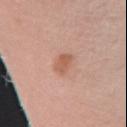Notes:
- subject — female, aged 48–52
- imaging modality — total-body-photography crop, ~15 mm field of view
- site — the left forearm
- lesion diameter — ≈3.5 mm
- lighting — white-light illumination
- automated lesion analysis — internal color variation of about 2.5 on a 0–10 scale